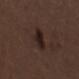Part of a total-body skin-imaging series; this lesion was reviewed on a skin check and was not flagged for biopsy. Automated image analysis of the tile measured an average lesion color of about L≈21 a*≈14 b*≈17 (CIELAB) and a lesion-to-skin contrast of about 10 (normalized; higher = more distinct). The analysis additionally found a color-variation rating of about 6.5/10 and a peripheral color-asymmetry measure near 2.5. On the chest. The patient is a female aged around 50. A roughly 15 mm field-of-view crop from a total-body skin photograph.